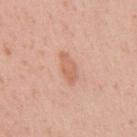Recorded during total-body skin imaging; not selected for excision or biopsy.
A male patient, aged approximately 55.
Cropped from a total-body skin-imaging series; the visible field is about 15 mm.
From the abdomen.